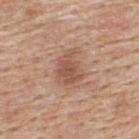Imaged during a routine full-body skin examination; the lesion was not biopsied and no histopathology is available.
From the back.
The lesion-visualizer software estimated an average lesion color of about L≈53 a*≈22 b*≈28 (CIELAB) and a normalized border contrast of about 7. The software also gave a border-irregularity rating of about 2.5/10, a color-variation rating of about 2.5/10, and radial color variation of about 1.
The recorded lesion diameter is about 3.5 mm.
A male subject, aged around 60.
This is a white-light tile.
This image is a 15 mm lesion crop taken from a total-body photograph.